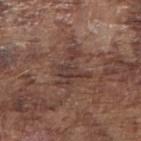Case summary:
* workup — total-body-photography surveillance lesion; no biopsy
* illumination — white-light illumination
* lesion diameter — ~4.5 mm (longest diameter)
* patient — male, about 75 years old
* image source — total-body-photography crop, ~15 mm field of view
* body site — the right upper arm